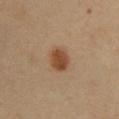  biopsy_status: not biopsied; imaged during a skin examination
  lighting: cross-polarized
  image:
    source: total-body photography crop
    field_of_view_mm: 15
  automated_metrics:
    border_irregularity_0_10: 1.0
    color_variation_0_10: 2.5
    peripheral_color_asymmetry: 1.0
    nevus_likeness_0_100: 100
  patient:
    sex: male
    age_approx: 50
  site: abdomen